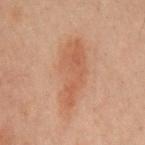Acquisition and patient details: A male patient, approximately 60 years of age. Automated image analysis of the tile measured an average lesion color of about L≈43 a*≈18 b*≈26 (CIELAB), about 6 CIELAB-L* units darker than the surrounding skin, and a normalized lesion–skin contrast near 5.5. The analysis additionally found a border-irregularity rating of about 4/10 and a color-variation rating of about 2.5/10. The analysis additionally found a classifier nevus-likeness of about 95/100 and lesion-presence confidence of about 100/100. Captured under cross-polarized illumination. Located on the chest. About 8 mm across. Cropped from a total-body skin-imaging series; the visible field is about 15 mm.